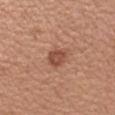{
  "biopsy_status": "not biopsied; imaged during a skin examination",
  "site": "left forearm",
  "image": {
    "source": "total-body photography crop",
    "field_of_view_mm": 15
  },
  "patient": {
    "sex": "female",
    "age_approx": 35
  },
  "lighting": "white-light",
  "lesion_size": {
    "long_diameter_mm_approx": 3.0
  }
}